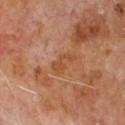Notes:
- workup — no biopsy performed (imaged during a skin exam)
- patient — male, about 65 years old
- anatomic site — the chest
- lighting — cross-polarized illumination
- image — ~15 mm crop, total-body skin-cancer survey
- TBP lesion metrics — an area of roughly 4 mm², a shape eccentricity near 0.85, and a shape-asymmetry score of about 0.5 (0 = symmetric); a lesion color around L≈47 a*≈24 b*≈36 in CIELAB and a lesion–skin lightness drop of about 6; a nevus-likeness score of about 0/100 and a detector confidence of about 100 out of 100 that the crop contains a lesion
- size — ~3 mm (longest diameter)